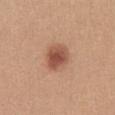* biopsy status: imaged on a skin check; not biopsied
* patient: female, aged 18–22
* diameter: ≈3.5 mm
* image: ~15 mm tile from a whole-body skin photo
* tile lighting: white-light
* site: the chest